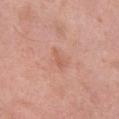Notes:
• follow-up · imaged on a skin check; not biopsied
• body site · the leg
• patient · female, approximately 50 years of age
• image source · 15 mm crop, total-body photography